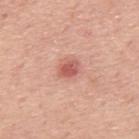Assessment: Part of a total-body skin-imaging series; this lesion was reviewed on a skin check and was not flagged for biopsy. Clinical summary: A roughly 15 mm field-of-view crop from a total-body skin photograph. A male patient aged approximately 75. Automated image analysis of the tile measured a nevus-likeness score of about 35/100 and a lesion-detection confidence of about 100/100. Imaged with white-light lighting. On the back. The recorded lesion diameter is about 2.5 mm.